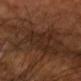Notes:
– biopsy status · catalogued during a skin exam; not biopsied
– lesion size · ~4.5 mm (longest diameter)
– subject · male, in their 60s
– location · the head or neck
– tile lighting · cross-polarized illumination
– acquisition · 15 mm crop, total-body photography